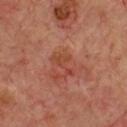  biopsy_status: not biopsied; imaged during a skin examination
  image:
    source: total-body photography crop
    field_of_view_mm: 15
  site: chest
  lighting: cross-polarized
  automated_metrics:
    area_mm2_approx: 4.5
    eccentricity: 0.85
    shape_asymmetry: 0.6
    cielab_L: 43
    cielab_a: 27
    cielab_b: 30
    vs_skin_darker_L: 6.0
    vs_skin_contrast_norm: 5.5
    color_variation_0_10: 1.5
    peripheral_color_asymmetry: 0.5
    nevus_likeness_0_100: 0
  lesion_size:
    long_diameter_mm_approx: 3.5
  patient:
    sex: male
    age_approx: 70